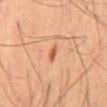The lesion was tiled from a total-body skin photograph and was not biopsied.
From the mid back.
Imaged with cross-polarized lighting.
An algorithmic analysis of the crop reported an automated nevus-likeness rating near 95 out of 100 and a lesion-detection confidence of about 100/100.
The patient is a male about 65 years old.
A roughly 15 mm field-of-view crop from a total-body skin photograph.
The lesion's longest dimension is about 2 mm.